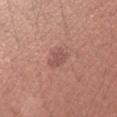This lesion was catalogued during total-body skin photography and was not selected for biopsy.
The lesion is on the left forearm.
The lesion-visualizer software estimated an automated nevus-likeness rating near 0 out of 100 and a lesion-detection confidence of about 100/100.
The patient is a female aged 23 to 27.
The lesion's longest dimension is about 2.5 mm.
Imaged with white-light lighting.
A roughly 15 mm field-of-view crop from a total-body skin photograph.